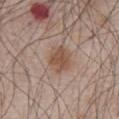Part of a total-body skin-imaging series; this lesion was reviewed on a skin check and was not flagged for biopsy. A male subject, in their mid-60s. On the front of the torso. The lesion's longest dimension is about 3.5 mm. Cropped from a whole-body photographic skin survey; the tile spans about 15 mm. Automated tile analysis of the lesion measured an area of roughly 7.5 mm² and a shape eccentricity near 0.65. The analysis additionally found an average lesion color of about L≈51 a*≈17 b*≈28 (CIELAB) and a lesion–skin lightness drop of about 9. It also reported a detector confidence of about 100 out of 100 that the crop contains a lesion. The tile uses white-light illumination.